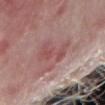Part of a total-body skin-imaging series; this lesion was reviewed on a skin check and was not flagged for biopsy. A male subject aged 73 to 77. A lesion tile, about 15 mm wide, cut from a 3D total-body photograph. The lesion is located on the right forearm.